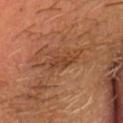notes: no biopsy performed (imaged during a skin exam)
subject: male, in their 40s
image-analysis metrics: a mean CIELAB color near L≈42 a*≈23 b*≈34, about 8 CIELAB-L* units darker than the surrounding skin, and a normalized border contrast of about 6; a lesion-detection confidence of about 65/100
lighting: cross-polarized illumination
image: 15 mm crop, total-body photography
size: ~3.5 mm (longest diameter)
anatomic site: the head or neck